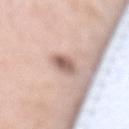Case summary:
– notes — total-body-photography surveillance lesion; no biopsy
– patient — male, aged around 80
– TBP lesion metrics — a footprint of about 4.5 mm², a shape eccentricity near 0.5, and two-axis asymmetry of about 0.15
– lighting — white-light
– location — the mid back
– acquisition — 15 mm crop, total-body photography
– size — ~2.5 mm (longest diameter)